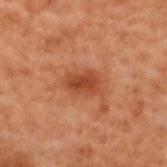Recorded during total-body skin imaging; not selected for excision or biopsy. A male patient, roughly 70 years of age. From the back. A 15 mm close-up extracted from a 3D total-body photography capture.